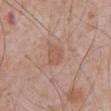Recorded during total-body skin imaging; not selected for excision or biopsy.